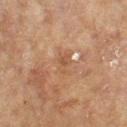Notes:
- biopsy status: catalogued during a skin exam; not biopsied
- lesion size: about 2.5 mm
- body site: the left forearm
- tile lighting: cross-polarized illumination
- subject: female, in their 80s
- automated metrics: an automated nevus-likeness rating near 0 out of 100 and a detector confidence of about 85 out of 100 that the crop contains a lesion
- acquisition: ~15 mm tile from a whole-body skin photo